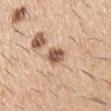Recorded during total-body skin imaging; not selected for excision or biopsy. The tile uses white-light illumination. The subject is a male aged 58 to 62. Approximately 3 mm at its widest. The lesion is on the right upper arm. Cropped from a total-body skin-imaging series; the visible field is about 15 mm.